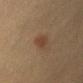Clinical impression: The lesion was tiled from a total-body skin photograph and was not biopsied. Background: An algorithmic analysis of the crop reported an area of roughly 4 mm², an outline eccentricity of about 0.75 (0 = round, 1 = elongated), and a symmetry-axis asymmetry near 0.25. It also reported an average lesion color of about L≈33 a*≈15 b*≈25 (CIELAB), about 6 CIELAB-L* units darker than the surrounding skin, and a normalized border contrast of about 6. And it measured a border-irregularity index near 2/10, a color-variation rating of about 2/10, and peripheral color asymmetry of about 0.5. The software also gave lesion-presence confidence of about 100/100. From the left upper arm. A female patient in their mid-50s. A lesion tile, about 15 mm wide, cut from a 3D total-body photograph. This is a cross-polarized tile.